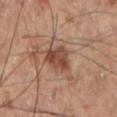* workup: imaged on a skin check; not biopsied
* subject: male, in their 60s
* lesion diameter: ≈3.5 mm
* image: ~15 mm tile from a whole-body skin photo
* site: the leg